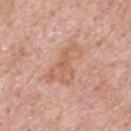Part of a total-body skin-imaging series; this lesion was reviewed on a skin check and was not flagged for biopsy.
A male patient, aged 53 to 57.
Located on the upper back.
Cropped from a total-body skin-imaging series; the visible field is about 15 mm.